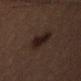Assessment:
Imaged during a routine full-body skin examination; the lesion was not biopsied and no histopathology is available.
Image and clinical context:
A female patient about 50 years old. Automated tile analysis of the lesion measured a lesion color around L≈14 a*≈11 b*≈14 in CIELAB and a lesion–skin lightness drop of about 7. The analysis additionally found a classifier nevus-likeness of about 95/100 and a lesion-detection confidence of about 100/100. The lesion is on the left thigh. Approximately 4.5 mm at its widest. A lesion tile, about 15 mm wide, cut from a 3D total-body photograph.